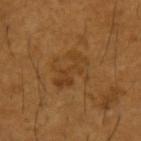This lesion was catalogued during total-body skin photography and was not selected for biopsy. Captured under cross-polarized illumination. An algorithmic analysis of the crop reported a shape eccentricity near 0.85 and a shape-asymmetry score of about 0.35 (0 = symmetric). The software also gave a border-irregularity index near 6/10. The lesion is located on the left upper arm. A male patient aged 63 to 67. The recorded lesion diameter is about 4.5 mm. A region of skin cropped from a whole-body photographic capture, roughly 15 mm wide.